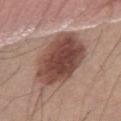Imaged during a routine full-body skin examination; the lesion was not biopsied and no histopathology is available. The recorded lesion diameter is about 8 mm. The lesion is on the abdomen. The tile uses white-light illumination. A male subject, aged approximately 35. Automated tile analysis of the lesion measured an average lesion color of about L≈46 a*≈20 b*≈24 (CIELAB) and a normalized lesion–skin contrast near 11. And it measured peripheral color asymmetry of about 2. A lesion tile, about 15 mm wide, cut from a 3D total-body photograph.